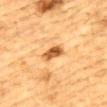Imaged during a routine full-body skin examination; the lesion was not biopsied and no histopathology is available. On the upper back. A male subject roughly 85 years of age or older. A region of skin cropped from a whole-body photographic capture, roughly 15 mm wide.